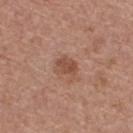Notes:
- body site · the upper back
- lesion size · about 3 mm
- subject · female, in their mid-60s
- lighting · white-light illumination
- image source · 15 mm crop, total-body photography